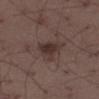{
  "lighting": "white-light",
  "patient": {
    "sex": "male",
    "age_approx": 40
  },
  "image": {
    "source": "total-body photography crop",
    "field_of_view_mm": 15
  },
  "lesion_size": {
    "long_diameter_mm_approx": 3.5
  },
  "automated_metrics": {
    "eccentricity": 0.5,
    "shape_asymmetry": 0.4,
    "cielab_L": 32,
    "cielab_a": 15,
    "cielab_b": 18,
    "vs_skin_darker_L": 8.0,
    "vs_skin_contrast_norm": 8.0,
    "border_irregularity_0_10": 4.0,
    "color_variation_0_10": 3.0,
    "peripheral_color_asymmetry": 1.0
  },
  "site": "right thigh"
}